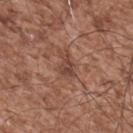Clinical impression: Captured during whole-body skin photography for melanoma surveillance; the lesion was not biopsied. Acquisition and patient details: A male subject, roughly 55 years of age. The recorded lesion diameter is about 3 mm. The lesion is located on the upper back. The lesion-visualizer software estimated an average lesion color of about L≈43 a*≈21 b*≈26 (CIELAB), about 8 CIELAB-L* units darker than the surrounding skin, and a lesion-to-skin contrast of about 7 (normalized; higher = more distinct). Imaged with white-light lighting. A roughly 15 mm field-of-view crop from a total-body skin photograph.